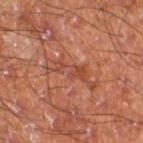notes — no biopsy performed (imaged during a skin exam)
anatomic site — the right thigh
tile lighting — cross-polarized illumination
image source — ~15 mm crop, total-body skin-cancer survey
image-analysis metrics — an area of roughly 9 mm², an outline eccentricity of about 0.95 (0 = round, 1 = elongated), and a symmetry-axis asymmetry near 0.5; an average lesion color of about L≈43 a*≈25 b*≈30 (CIELAB), about 7 CIELAB-L* units darker than the surrounding skin, and a lesion-to-skin contrast of about 5 (normalized; higher = more distinct); a border-irregularity index near 8.5/10, internal color variation of about 3 on a 0–10 scale, and a peripheral color-asymmetry measure near 0.5; a nevus-likeness score of about 0/100 and lesion-presence confidence of about 60/100
lesion size — ≈6 mm
patient — male, aged 58 to 62